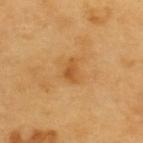notes — imaged on a skin check; not biopsied